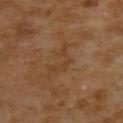Recorded during total-body skin imaging; not selected for excision or biopsy. This is a cross-polarized tile. Approximately 4 mm at its widest. An algorithmic analysis of the crop reported a lesion–skin lightness drop of about 5 and a lesion-to-skin contrast of about 4.5 (normalized; higher = more distinct). The analysis additionally found an automated nevus-likeness rating near 0 out of 100 and a detector confidence of about 100 out of 100 that the crop contains a lesion. A region of skin cropped from a whole-body photographic capture, roughly 15 mm wide. The subject is a female roughly 55 years of age. From the upper back.